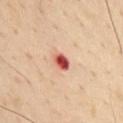  biopsy_status: not biopsied; imaged during a skin examination
  site: chest
  lighting: cross-polarized
  image:
    source: total-body photography crop
    field_of_view_mm: 15
  patient:
    sex: male
    age_approx: 55